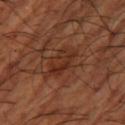The lesion was tiled from a total-body skin photograph and was not biopsied.
A 15 mm close-up extracted from a 3D total-body photography capture.
Longest diameter approximately 4 mm.
A male subject in their mid-60s.
The lesion is located on the right thigh.